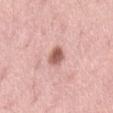No biopsy was performed on this lesion — it was imaged during a full skin examination and was not determined to be concerning. A region of skin cropped from a whole-body photographic capture, roughly 15 mm wide. The lesion is on the lower back. A female subject, roughly 50 years of age.